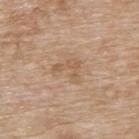The lesion was tiled from a total-body skin photograph and was not biopsied.
A close-up tile cropped from a whole-body skin photograph, about 15 mm across.
A female subject, aged 73–77.
About 3.5 mm across.
On the upper back.
This is a white-light tile.